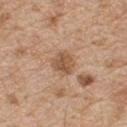Captured during whole-body skin photography for melanoma surveillance; the lesion was not biopsied.
The recorded lesion diameter is about 3 mm.
The tile uses white-light illumination.
A close-up tile cropped from a whole-body skin photograph, about 15 mm across.
On the upper back.
A male patient aged 68 to 72.